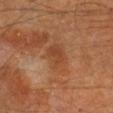Clinical impression: Captured during whole-body skin photography for melanoma surveillance; the lesion was not biopsied. Background: A male subject aged approximately 65. A roughly 15 mm field-of-view crop from a total-body skin photograph. About 4.5 mm across. Located on the right lower leg.